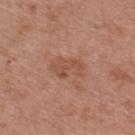follow-up = catalogued during a skin exam; not biopsied
subject = female, aged 38–42
body site = the upper back
lighting = white-light illumination
image = total-body-photography crop, ~15 mm field of view
lesion diameter = ~4 mm (longest diameter)